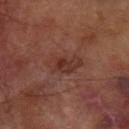{"biopsy_status": "not biopsied; imaged during a skin examination", "site": "right forearm", "automated_metrics": {"area_mm2_approx": 9.0, "eccentricity": 0.8, "shape_asymmetry": 0.2, "color_variation_0_10": 4.5, "peripheral_color_asymmetry": 1.5}, "image": {"source": "total-body photography crop", "field_of_view_mm": 15}, "lesion_size": {"long_diameter_mm_approx": 4.5}, "patient": {"sex": "male", "age_approx": 65}, "lighting": "cross-polarized"}